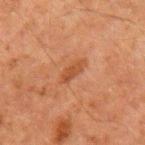Q: Is there a histopathology result?
A: imaged on a skin check; not biopsied
Q: Who is the patient?
A: male, aged around 60
Q: Lesion location?
A: the left upper arm
Q: How large is the lesion?
A: ~3.5 mm (longest diameter)
Q: What is the imaging modality?
A: ~15 mm tile from a whole-body skin photo
Q: How was the tile lit?
A: cross-polarized
Q: Automated lesion metrics?
A: a lesion color around L≈38 a*≈22 b*≈31 in CIELAB, a lesion–skin lightness drop of about 7, and a lesion-to-skin contrast of about 6.5 (normalized; higher = more distinct); a classifier nevus-likeness of about 5/100